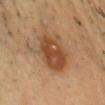Q: Is there a histopathology result?
A: no biopsy performed (imaged during a skin exam)
Q: How was the tile lit?
A: cross-polarized
Q: How large is the lesion?
A: ≈6 mm
Q: Where on the body is the lesion?
A: the chest
Q: What is the imaging modality?
A: ~15 mm tile from a whole-body skin photo
Q: Patient demographics?
A: male, in their mid- to late 40s